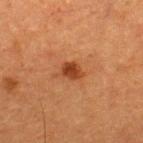Impression: Captured during whole-body skin photography for melanoma surveillance; the lesion was not biopsied. Image and clinical context: About 2.5 mm across. A 15 mm close-up extracted from a 3D total-body photography capture. On the upper back. Captured under cross-polarized illumination. A male patient roughly 50 years of age.